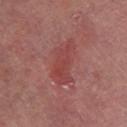The lesion was tiled from a total-body skin photograph and was not biopsied.
A 15 mm close-up tile from a total-body photography series done for melanoma screening.
The tile uses cross-polarized illumination.
The lesion is on the leg.
The subject is a male aged around 65.